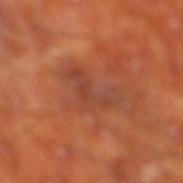Background: About 5.5 mm across. A 15 mm close-up extracted from a 3D total-body photography capture. A male patient roughly 65 years of age. Automated image analysis of the tile measured a lesion area of about 9 mm² and a shape-asymmetry score of about 0.65 (0 = symmetric). The analysis additionally found a lesion color around L≈41 a*≈26 b*≈31 in CIELAB and a lesion–skin lightness drop of about 6. The software also gave a border-irregularity index near 8.5/10, a within-lesion color-variation index near 4/10, and a peripheral color-asymmetry measure near 1.5. The lesion is located on the leg.